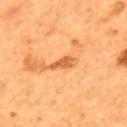The lesion was tiled from a total-body skin photograph and was not biopsied. Cropped from a whole-body photographic skin survey; the tile spans about 15 mm. On the upper back. The patient is a male approximately 55 years of age. The recorded lesion diameter is about 3 mm.